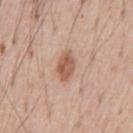<tbp_lesion>
  <biopsy_status>not biopsied; imaged during a skin examination</biopsy_status>
  <automated_metrics>
    <area_mm2_approx>6.0</area_mm2_approx>
    <eccentricity>0.8</eccentricity>
    <shape_asymmetry>0.15</shape_asymmetry>
    <color_variation_0_10>3.5</color_variation_0_10>
  </automated_metrics>
  <lesion_size>
    <long_diameter_mm_approx>3.5</long_diameter_mm_approx>
  </lesion_size>
  <lighting>white-light</lighting>
  <image>
    <source>total-body photography crop</source>
    <field_of_view_mm>15</field_of_view_mm>
  </image>
  <site>chest</site>
  <patient>
    <sex>male</sex>
    <age_approx>55</age_approx>
  </patient>
</tbp_lesion>